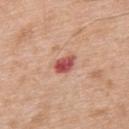No biopsy was performed on this lesion — it was imaged during a full skin examination and was not determined to be concerning. The lesion is located on the upper back. This image is a 15 mm lesion crop taken from a total-body photograph. A male patient, in their 50s. This is a white-light tile.